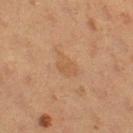site: leg
automated_metrics:
  border_irregularity_0_10: 3.0
  color_variation_0_10: 1.0
  peripheral_color_asymmetry: 0.5
lighting: cross-polarized
image:
  source: total-body photography crop
  field_of_view_mm: 15
lesion_size:
  long_diameter_mm_approx: 3.0
patient:
  sex: female
  age_approx: 20